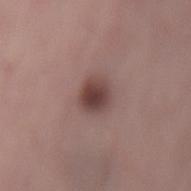Q: Was a biopsy performed?
A: imaged on a skin check; not biopsied
Q: Where on the body is the lesion?
A: the mid back
Q: Lesion size?
A: ≈3 mm
Q: Illumination type?
A: white-light illumination
Q: What kind of image is this?
A: 15 mm crop, total-body photography
Q: Patient demographics?
A: female, aged 63–67